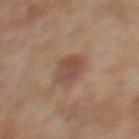Case summary:
- notes — no biopsy performed (imaged during a skin exam)
- TBP lesion metrics — a lesion color around L≈47 a*≈19 b*≈26 in CIELAB and about 8 CIELAB-L* units darker than the surrounding skin
- subject — female, aged approximately 50
- body site — the back
- illumination — cross-polarized illumination
- image — total-body-photography crop, ~15 mm field of view